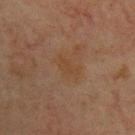Case summary:
* biopsy status · total-body-photography surveillance lesion; no biopsy
* site · the upper back
* imaging modality · 15 mm crop, total-body photography
* lighting · cross-polarized
* subject · male, in their 60s
* automated lesion analysis · a lesion area of about 4.5 mm², an outline eccentricity of about 0.85 (0 = round, 1 = elongated), and two-axis asymmetry of about 0.3
* lesion size · about 3 mm